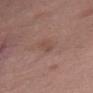Assessment: The lesion was photographed on a routine skin check and not biopsied; there is no pathology result. Clinical summary: A female patient aged approximately 40. From the abdomen. About 2.5 mm across. This image is a 15 mm lesion crop taken from a total-body photograph. Imaged with white-light lighting.